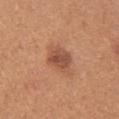biopsy status: catalogued during a skin exam; not biopsied | location: the upper back | subject: female, aged 38–42 | imaging modality: ~15 mm tile from a whole-body skin photo | lesion diameter: ~3.5 mm (longest diameter).